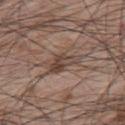Captured during whole-body skin photography for melanoma surveillance; the lesion was not biopsied. A male subject aged 63 to 67. The tile uses white-light illumination. A lesion tile, about 15 mm wide, cut from a 3D total-body photograph. From the upper back. The lesion's longest dimension is about 4.5 mm.